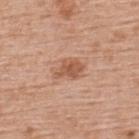Captured during whole-body skin photography for melanoma surveillance; the lesion was not biopsied. The lesion is on the upper back. Cropped from a total-body skin-imaging series; the visible field is about 15 mm. A female subject roughly 65 years of age.